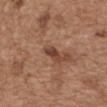workup: total-body-photography surveillance lesion; no biopsy
location: the chest
automated lesion analysis: a mean CIELAB color near L≈41 a*≈22 b*≈28 and a lesion–skin lightness drop of about 12; a border-irregularity index near 4/10, a within-lesion color-variation index near 0/10, and a peripheral color-asymmetry measure near 0; a nevus-likeness score of about 0/100 and a lesion-detection confidence of about 95/100
tile lighting: white-light illumination
patient: male, in their mid-50s
lesion size: ~3 mm (longest diameter)
image: 15 mm crop, total-body photography